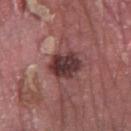biopsy_status: not biopsied; imaged during a skin examination
lesion_size:
  long_diameter_mm_approx: 4.5
image:
  source: total-body photography crop
  field_of_view_mm: 15
site: left lower leg
patient:
  sex: male
  age_approx: 40